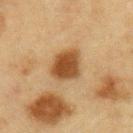{
  "site": "right upper arm",
  "image": {
    "source": "total-body photography crop",
    "field_of_view_mm": 15
  },
  "patient": {
    "sex": "male",
    "age_approx": 65
  }
}